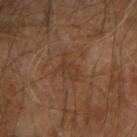This lesion was catalogued during total-body skin photography and was not selected for biopsy.
The recorded lesion diameter is about 4 mm.
Imaged with cross-polarized lighting.
The subject is a male aged approximately 70.
Located on the right upper arm.
A lesion tile, about 15 mm wide, cut from a 3D total-body photograph.